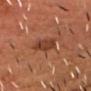  biopsy_status: not biopsied; imaged during a skin examination
  patient:
    sex: male
    age_approx: 55
  site: head or neck
  lighting: cross-polarized
  image:
    source: total-body photography crop
    field_of_view_mm: 15
  lesion_size:
    long_diameter_mm_approx: 2.5